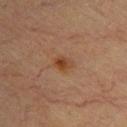biopsy status: catalogued during a skin exam; not biopsied | body site: the upper back | subject: female, aged around 65 | image: ~15 mm crop, total-body skin-cancer survey | diameter: ~2.5 mm (longest diameter) | automated lesion analysis: an area of roughly 3.5 mm² and an eccentricity of roughly 0.75; a mean CIELAB color near L≈36 a*≈19 b*≈30, about 7 CIELAB-L* units darker than the surrounding skin, and a normalized lesion–skin contrast near 8; internal color variation of about 5 on a 0–10 scale and radial color variation of about 1.5 | tile lighting: cross-polarized illumination.